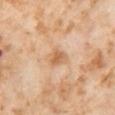- biopsy status · catalogued during a skin exam; not biopsied
- size · about 2.5 mm
- imaging modality · 15 mm crop, total-body photography
- patient · female, roughly 55 years of age
- location · the left thigh